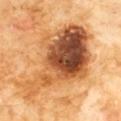workup = catalogued during a skin exam; not biopsied | TBP lesion metrics = a lesion color around L≈52 a*≈24 b*≈40 in CIELAB, a lesion–skin lightness drop of about 18, and a normalized lesion–skin contrast near 11.5; border irregularity of about 6 on a 0–10 scale and a within-lesion color-variation index near 10/10; a nevus-likeness score of about 0/100 and a detector confidence of about 100 out of 100 that the crop contains a lesion | tile lighting = cross-polarized | subject = male, in their 60s | imaging modality = ~15 mm crop, total-body skin-cancer survey | anatomic site = the chest.